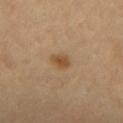workup = total-body-photography surveillance lesion; no biopsy | image source = total-body-photography crop, ~15 mm field of view | size = ≈2 mm | illumination = cross-polarized | patient = aged 63–67 | location = the mid back.